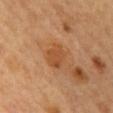Part of a total-body skin-imaging series; this lesion was reviewed on a skin check and was not flagged for biopsy. Located on the mid back. Captured under cross-polarized illumination. A close-up tile cropped from a whole-body skin photograph, about 15 mm across. A female subject, in their 60s. Approximately 3 mm at its widest.